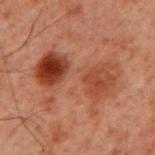biopsy status = total-body-photography surveillance lesion; no biopsy | diameter = ≈9 mm | image source = ~15 mm crop, total-body skin-cancer survey | anatomic site = the mid back | patient = male, in their 60s.